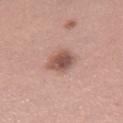Captured during whole-body skin photography for melanoma surveillance; the lesion was not biopsied. The lesion is on the leg. The patient is a female roughly 30 years of age. This image is a 15 mm lesion crop taken from a total-body photograph.